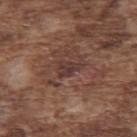follow-up = imaged on a skin check; not biopsied | lesion diameter = about 3 mm | automated metrics = a footprint of about 4.5 mm², a shape eccentricity near 0.8, and a symmetry-axis asymmetry near 0.4; a color-variation rating of about 2/10 and a peripheral color-asymmetry measure near 1 | subject = male, approximately 75 years of age | image = ~15 mm crop, total-body skin-cancer survey | site = the upper back | lighting = white-light illumination.